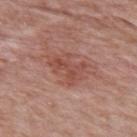A roughly 15 mm field-of-view crop from a total-body skin photograph. Automated image analysis of the tile measured a footprint of about 11 mm², a shape eccentricity near 0.75, and a shape-asymmetry score of about 0.35 (0 = symmetric). And it measured a nevus-likeness score of about 5/100 and a detector confidence of about 100 out of 100 that the crop contains a lesion. The patient is a female about 60 years old. The tile uses white-light illumination. The lesion is located on the upper back.